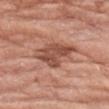biopsy status — no biopsy performed (imaged during a skin exam); acquisition — ~15 mm tile from a whole-body skin photo; site — the arm; lesion diameter — ~4.5 mm (longest diameter); patient — female, aged around 75; illumination — white-light.